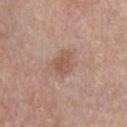The lesion was photographed on a routine skin check and not biopsied; there is no pathology result.
The recorded lesion diameter is about 3 mm.
A male patient aged 58 to 62.
On the chest.
The tile uses white-light illumination.
A 15 mm crop from a total-body photograph taken for skin-cancer surveillance.